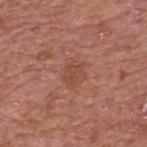Recorded during total-body skin imaging; not selected for excision or biopsy. A 15 mm crop from a total-body photograph taken for skin-cancer surveillance. About 2.5 mm across. From the upper back. Automated tile analysis of the lesion measured a lesion area of about 4 mm², an outline eccentricity of about 0.6 (0 = round, 1 = elongated), and a shape-asymmetry score of about 0.3 (0 = symmetric). It also reported a nevus-likeness score of about 0/100 and a lesion-detection confidence of about 100/100. Captured under white-light illumination. A male patient aged 63 to 67.